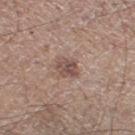<record>
<biopsy_status>not biopsied; imaged during a skin examination</biopsy_status>
</record>